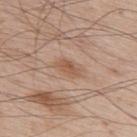Recorded during total-body skin imaging; not selected for excision or biopsy. About 3 mm across. Automated tile analysis of the lesion measured an area of roughly 3.5 mm², an outline eccentricity of about 0.85 (0 = round, 1 = elongated), and a symmetry-axis asymmetry near 0.35. The analysis additionally found a border-irregularity rating of about 3.5/10, internal color variation of about 2.5 on a 0–10 scale, and a peripheral color-asymmetry measure near 1. And it measured an automated nevus-likeness rating near 0 out of 100 and lesion-presence confidence of about 100/100. A 15 mm crop from a total-body photograph taken for skin-cancer surveillance. The patient is a male aged 63 to 67. The tile uses white-light illumination. The lesion is located on the back.